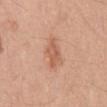Imaged during a routine full-body skin examination; the lesion was not biopsied and no histopathology is available. A close-up tile cropped from a whole-body skin photograph, about 15 mm across. The total-body-photography lesion software estimated lesion-presence confidence of about 100/100. On the mid back. Approximately 3.5 mm at its widest. A male patient, in their mid- to late 40s. The tile uses white-light illumination.